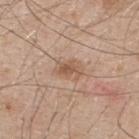<case>
  <biopsy_status>not biopsied; imaged during a skin examination</biopsy_status>
  <patient>
    <sex>male</sex>
    <age_approx>50</age_approx>
  </patient>
  <lighting>white-light</lighting>
  <lesion_size>
    <long_diameter_mm_approx>3.5</long_diameter_mm_approx>
  </lesion_size>
  <image>
    <source>total-body photography crop</source>
    <field_of_view_mm>15</field_of_view_mm>
  </image>
  <site>upper back</site>
  <automated_metrics>
    <area_mm2_approx>5.5</area_mm2_approx>
    <eccentricity>0.75</eccentricity>
    <shape_asymmetry>0.3</shape_asymmetry>
    <vs_skin_darker_L>9.0</vs_skin_darker_L>
    <vs_skin_contrast_norm>6.5</vs_skin_contrast_norm>
    <nevus_likeness_0_100>55</nevus_likeness_0_100>
    <lesion_detection_confidence_0_100>100</lesion_detection_confidence_0_100>
  </automated_metrics>
</case>